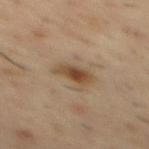Part of a total-body skin-imaging series; this lesion was reviewed on a skin check and was not flagged for biopsy.
The recorded lesion diameter is about 3.5 mm.
The lesion is located on the back.
The subject is a male aged around 55.
A 15 mm close-up tile from a total-body photography series done for melanoma screening.
Automated image analysis of the tile measured an area of roughly 5.5 mm². It also reported an average lesion color of about L≈44 a*≈17 b*≈31 (CIELAB) and a normalized lesion–skin contrast near 9.5.